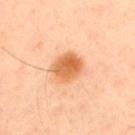| field | value |
|---|---|
| anatomic site | the upper back |
| imaging modality | ~15 mm tile from a whole-body skin photo |
| subject | male, about 45 years old |
| lesion diameter | about 4 mm |